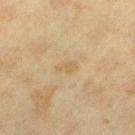{"biopsy_status": "not biopsied; imaged during a skin examination", "image": {"source": "total-body photography crop", "field_of_view_mm": 15}, "lesion_size": {"long_diameter_mm_approx": 2.5}, "lighting": "cross-polarized", "site": "leg", "patient": {"sex": "female", "age_approx": 40}}